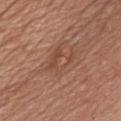| field | value |
|---|---|
| location | the chest |
| image source | ~15 mm crop, total-body skin-cancer survey |
| patient | male, approximately 60 years of age |